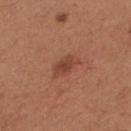The lesion is on the back.
Longest diameter approximately 3.5 mm.
Captured under white-light illumination.
A 15 mm close-up extracted from a 3D total-body photography capture.
A female subject approximately 30 years of age.